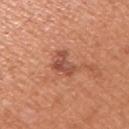Part of a total-body skin-imaging series; this lesion was reviewed on a skin check and was not flagged for biopsy. This image is a 15 mm lesion crop taken from a total-body photograph. About 3.5 mm across. A male subject in their mid- to late 50s. Automated image analysis of the tile measured a mean CIELAB color near L≈51 a*≈27 b*≈31, a lesion–skin lightness drop of about 10, and a lesion-to-skin contrast of about 7.5 (normalized; higher = more distinct). On the right upper arm.